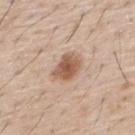Recorded during total-body skin imaging; not selected for excision or biopsy.
Located on the upper back.
Imaged with white-light lighting.
A 15 mm crop from a total-body photograph taken for skin-cancer surveillance.
The subject is a male in their mid- to late 50s.